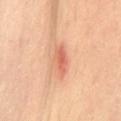Q: Was this lesion biopsied?
A: catalogued during a skin exam; not biopsied
Q: How was this image acquired?
A: ~15 mm tile from a whole-body skin photo
Q: What did automated image analysis measure?
A: a footprint of about 6.5 mm² and an eccentricity of roughly 0.9; a lesion color around L≈65 a*≈28 b*≈35 in CIELAB, a lesion–skin lightness drop of about 11, and a lesion-to-skin contrast of about 6.5 (normalized; higher = more distinct); a border-irregularity index near 2.5/10 and peripheral color asymmetry of about 1.5
Q: Lesion location?
A: the mid back
Q: How was the tile lit?
A: cross-polarized
Q: Lesion size?
A: about 4 mm
Q: Patient demographics?
A: female, in their 40s